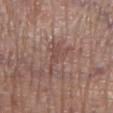patient — male, aged 78 to 82
image source — total-body-photography crop, ~15 mm field of view
illumination — white-light
diameter — about 4.5 mm
site — the left lower leg
TBP lesion metrics — about 6 CIELAB-L* units darker than the surrounding skin and a normalized border contrast of about 5; a detector confidence of about 70 out of 100 that the crop contains a lesion